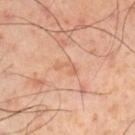Captured during whole-body skin photography for melanoma surveillance; the lesion was not biopsied. The subject is a male in their mid- to late 50s. This is a cross-polarized tile. The lesion is located on the right lower leg. Cropped from a total-body skin-imaging series; the visible field is about 15 mm.